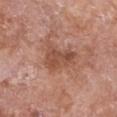{"biopsy_status": "not biopsied; imaged during a skin examination", "image": {"source": "total-body photography crop", "field_of_view_mm": 15}, "lighting": "white-light", "site": "chest", "patient": {"sex": "female", "age_approx": 75}, "lesion_size": {"long_diameter_mm_approx": 4.0}, "automated_metrics": {"eccentricity": 0.7, "cielab_L": 50, "cielab_a": 23, "cielab_b": 29, "vs_skin_darker_L": 9.0, "vs_skin_contrast_norm": 7.0, "border_irregularity_0_10": 4.5, "color_variation_0_10": 3.5, "peripheral_color_asymmetry": 1.5}}